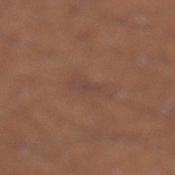Case summary:
– follow-up · total-body-photography surveillance lesion; no biopsy
– site · the leg
– subject · male, aged 43 to 47
– image-analysis metrics · a lesion area of about 4.5 mm², an outline eccentricity of about 0.95 (0 = round, 1 = elongated), and a symmetry-axis asymmetry near 0.35; a mean CIELAB color near L≈43 a*≈18 b*≈25 and a lesion-to-skin contrast of about 4.5 (normalized; higher = more distinct)
– acquisition · ~15 mm crop, total-body skin-cancer survey